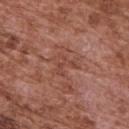Case summary:
• follow-up — total-body-photography surveillance lesion; no biopsy
• lighting — white-light
• image source — total-body-photography crop, ~15 mm field of view
• location — the upper back
• subject — male, aged around 75
• size — ~3 mm (longest diameter)